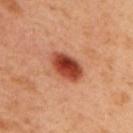Case summary:
- notes · imaged on a skin check; not biopsied
- tile lighting · cross-polarized illumination
- body site · the upper back
- subject · male, in their 50s
- image-analysis metrics · a border-irregularity rating of about 1.5/10; a nevus-likeness score of about 100/100 and a lesion-detection confidence of about 100/100
- lesion size · ≈4.5 mm
- image · ~15 mm crop, total-body skin-cancer survey